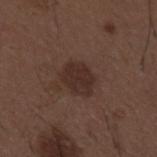The lesion is on the mid back.
The patient is a male approximately 50 years of age.
This image is a 15 mm lesion crop taken from a total-body photograph.